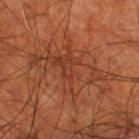No biopsy was performed on this lesion — it was imaged during a full skin examination and was not determined to be concerning. A region of skin cropped from a whole-body photographic capture, roughly 15 mm wide. Located on the right thigh. A male patient aged 68–72.